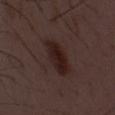Captured during whole-body skin photography for melanoma surveillance; the lesion was not biopsied.
Captured under white-light illumination.
Approximately 6 mm at its widest.
A 15 mm close-up extracted from a 3D total-body photography capture.
A male patient, roughly 50 years of age.